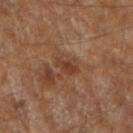The lesion was photographed on a routine skin check and not biopsied; there is no pathology result.
The patient is a male approximately 60 years of age.
Located on the right forearm.
A 15 mm close-up extracted from a 3D total-body photography capture.
This is a cross-polarized tile.
Automated tile analysis of the lesion measured an average lesion color of about L≈37 a*≈20 b*≈29 (CIELAB) and about 8 CIELAB-L* units darker than the surrounding skin. And it measured a within-lesion color-variation index near 1/10.
The recorded lesion diameter is about 3 mm.